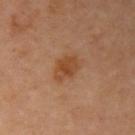Clinical impression: This lesion was catalogued during total-body skin photography and was not selected for biopsy. Context: The lesion is on the right upper arm. The patient is a female approximately 60 years of age. The lesion's longest dimension is about 3.5 mm. Cropped from a total-body skin-imaging series; the visible field is about 15 mm.